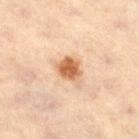Image and clinical context:
The lesion's longest dimension is about 3 mm. A close-up tile cropped from a whole-body skin photograph, about 15 mm across. Located on the right thigh. A male patient, about 60 years old. Automated image analysis of the tile measured a lesion area of about 6 mm², a shape eccentricity near 0.55, and a symmetry-axis asymmetry near 0.2. The software also gave a border-irregularity rating of about 1.5/10.